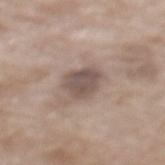{"lighting": "white-light", "image": {"source": "total-body photography crop", "field_of_view_mm": 15}, "automated_metrics": {"area_mm2_approx": 8.0, "eccentricity": 0.3, "shape_asymmetry": 0.25, "cielab_L": 51, "cielab_a": 14, "cielab_b": 21, "vs_skin_darker_L": 11.0, "nevus_likeness_0_100": 5}, "lesion_size": {"long_diameter_mm_approx": 3.5}, "site": "back", "patient": {"sex": "female", "age_approx": 75}}